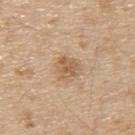A roughly 15 mm field-of-view crop from a total-body skin photograph.
Automated image analysis of the tile measured an area of roughly 5 mm², an eccentricity of roughly 0.4, and a shape-asymmetry score of about 0.4 (0 = symmetric). The analysis additionally found an average lesion color of about L≈58 a*≈18 b*≈34 (CIELAB), a lesion–skin lightness drop of about 10, and a lesion-to-skin contrast of about 6.5 (normalized; higher = more distinct). The software also gave border irregularity of about 4 on a 0–10 scale, internal color variation of about 3 on a 0–10 scale, and radial color variation of about 1.
Imaged with white-light lighting.
Located on the upper back.
About 2.5 mm across.
A male subject, aged approximately 70.